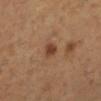Assessment:
Recorded during total-body skin imaging; not selected for excision or biopsy.
Background:
The lesion is on the left lower leg. The tile uses cross-polarized illumination. The total-body-photography lesion software estimated a classifier nevus-likeness of about 90/100 and a lesion-detection confidence of about 100/100. The lesion's longest dimension is about 2 mm. The patient is a female roughly 55 years of age. A 15 mm crop from a total-body photograph taken for skin-cancer surveillance.